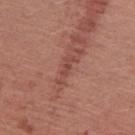biopsy status: imaged on a skin check; not biopsied | acquisition: ~15 mm tile from a whole-body skin photo | patient: female, aged around 50 | illumination: white-light illumination | lesion size: ~4 mm (longest diameter) | body site: the right upper arm | TBP lesion metrics: an eccentricity of roughly 0.95.